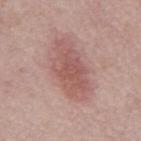Notes:
• biopsy status — imaged on a skin check; not biopsied
• subject — male, about 65 years old
• TBP lesion metrics — a footprint of about 19 mm² and a shape-asymmetry score of about 0.2 (0 = symmetric); a lesion color around L≈55 a*≈23 b*≈23 in CIELAB and a lesion-to-skin contrast of about 6.5 (normalized; higher = more distinct); border irregularity of about 3 on a 0–10 scale, a color-variation rating of about 3/10, and peripheral color asymmetry of about 1; a detector confidence of about 100 out of 100 that the crop contains a lesion
• imaging modality — total-body-photography crop, ~15 mm field of view
• site — the back